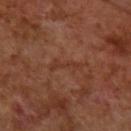workup=imaged on a skin check; not biopsied | anatomic site=the right forearm | imaging modality=15 mm crop, total-body photography | patient=female, aged 63 to 67.